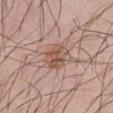biopsy status — no biopsy performed (imaged during a skin exam) | image — ~15 mm crop, total-body skin-cancer survey | automated metrics — a lesion area of about 7.5 mm², an outline eccentricity of about 0.65 (0 = round, 1 = elongated), and a symmetry-axis asymmetry near 0.2; roughly 10 lightness units darker than nearby skin and a normalized lesion–skin contrast near 7 | lesion size — ≈3.5 mm | location — the front of the torso | patient — male, about 25 years old | tile lighting — white-light.